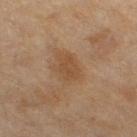Imaged during a routine full-body skin examination; the lesion was not biopsied and no histopathology is available. Captured under cross-polarized illumination. Longest diameter approximately 3 mm. The total-body-photography lesion software estimated an area of roughly 5 mm² and a shape eccentricity near 0.75. And it measured a lesion color around L≈43 a*≈17 b*≈31 in CIELAB. The software also gave a classifier nevus-likeness of about 15/100 and a detector confidence of about 100 out of 100 that the crop contains a lesion. A 15 mm close-up tile from a total-body photography series done for melanoma screening. A female patient, roughly 60 years of age. Located on the right thigh.